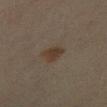| field | value |
|---|---|
| follow-up | total-body-photography surveillance lesion; no biopsy |
| lesion diameter | ~3 mm (longest diameter) |
| automated lesion analysis | a lesion area of about 4.5 mm², an outline eccentricity of about 0.7 (0 = round, 1 = elongated), and a symmetry-axis asymmetry near 0.3; a mean CIELAB color near L≈30 a*≈11 b*≈21, a lesion–skin lightness drop of about 6, and a normalized border contrast of about 8; border irregularity of about 2.5 on a 0–10 scale, a color-variation rating of about 1.5/10, and radial color variation of about 0.5; a classifier nevus-likeness of about 75/100 and lesion-presence confidence of about 100/100 |
| body site | the right lower leg |
| image | ~15 mm crop, total-body skin-cancer survey |
| tile lighting | cross-polarized illumination |
| subject | female, approximately 45 years of age |